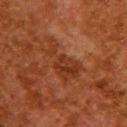This lesion was catalogued during total-body skin photography and was not selected for biopsy. The patient is a female aged around 50. Imaged with cross-polarized lighting. A close-up tile cropped from a whole-body skin photograph, about 15 mm across. The lesion is on the upper back.